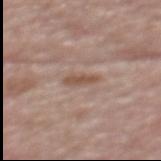Assessment: This lesion was catalogued during total-body skin photography and was not selected for biopsy. Image and clinical context: A roughly 15 mm field-of-view crop from a total-body skin photograph. Located on the back. Longest diameter approximately 3.5 mm. A male patient approximately 80 years of age.